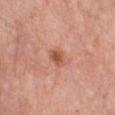biopsy status: catalogued during a skin exam; not biopsied
body site: the chest
subject: female, in their mid-50s
imaging modality: ~15 mm tile from a whole-body skin photo